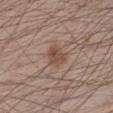follow-up: total-body-photography surveillance lesion; no biopsy | subject: male, approximately 35 years of age | imaging modality: total-body-photography crop, ~15 mm field of view | body site: the left lower leg.